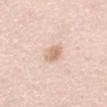A female patient aged approximately 30. Approximately 3 mm at its widest. A region of skin cropped from a whole-body photographic capture, roughly 15 mm wide. This is a white-light tile. The lesion is on the abdomen. The total-body-photography lesion software estimated roughly 10 lightness units darker than nearby skin. The software also gave a nevus-likeness score of about 40/100 and lesion-presence confidence of about 100/100.